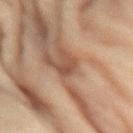The lesion was photographed on a routine skin check and not biopsied; there is no pathology result. A close-up tile cropped from a whole-body skin photograph, about 15 mm across. This is a cross-polarized tile. Located on the right forearm. A female patient, aged approximately 80.